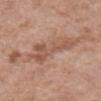Q: Was this lesion biopsied?
A: no biopsy performed (imaged during a skin exam)
Q: What is the anatomic site?
A: the front of the torso
Q: What kind of image is this?
A: 15 mm crop, total-body photography
Q: Patient demographics?
A: male, roughly 70 years of age
Q: What is the lesion's diameter?
A: ~5.5 mm (longest diameter)
Q: What did automated image analysis measure?
A: a border-irregularity rating of about 9/10, internal color variation of about 3 on a 0–10 scale, and a peripheral color-asymmetry measure near 0.5; an automated nevus-likeness rating near 0 out of 100 and a lesion-detection confidence of about 100/100